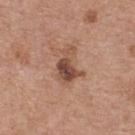Case summary:
– notes — imaged on a skin check; not biopsied
– tile lighting — white-light
– location — the upper back
– subject — male, aged around 80
– lesion size — ~4 mm (longest diameter)
– TBP lesion metrics — an area of roughly 8.5 mm², an outline eccentricity of about 0.75 (0 = round, 1 = elongated), and a shape-asymmetry score of about 0.45 (0 = symmetric); an average lesion color of about L≈49 a*≈21 b*≈28 (CIELAB), about 12 CIELAB-L* units darker than the surrounding skin, and a lesion-to-skin contrast of about 8.5 (normalized; higher = more distinct); a classifier nevus-likeness of about 55/100 and a detector confidence of about 100 out of 100 that the crop contains a lesion
– acquisition — ~15 mm crop, total-body skin-cancer survey